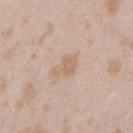Q: Was this lesion biopsied?
A: imaged on a skin check; not biopsied
Q: Who is the patient?
A: female, aged approximately 25
Q: Illumination type?
A: white-light
Q: Where on the body is the lesion?
A: the left thigh
Q: What is the imaging modality?
A: 15 mm crop, total-body photography
Q: What is the lesion's diameter?
A: ≈3.5 mm